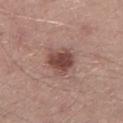Findings:
* biopsy status · catalogued during a skin exam; not biopsied